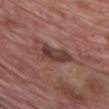Context:
The lesion is located on the chest. A 15 mm close-up extracted from a 3D total-body photography capture. The lesion's longest dimension is about 5 mm. Imaged with white-light lighting. The patient is a male in their mid-60s.